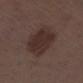This lesion was catalogued during total-body skin photography and was not selected for biopsy.
Automated image analysis of the tile measured border irregularity of about 1 on a 0–10 scale, a within-lesion color-variation index near 3/10, and a peripheral color-asymmetry measure near 1. It also reported a detector confidence of about 100 out of 100 that the crop contains a lesion.
About 5 mm across.
The patient is a male in their 70s.
This is a white-light tile.
The lesion is on the left thigh.
A 15 mm close-up extracted from a 3D total-body photography capture.